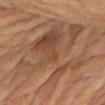<lesion>
<biopsy_status>not biopsied; imaged during a skin examination</biopsy_status>
<automated_metrics>
  <eccentricity>0.7</eccentricity>
  <cielab_L>46</cielab_L>
  <cielab_a>19</cielab_a>
  <cielab_b>30</cielab_b>
  <vs_skin_darker_L>7.0</vs_skin_darker_L>
  <vs_skin_contrast_norm>6.0</vs_skin_contrast_norm>
  <border_irregularity_0_10>9.0</border_irregularity_0_10>
  <color_variation_0_10>6.5</color_variation_0_10>
  <peripheral_color_asymmetry>2.0</peripheral_color_asymmetry>
  <nevus_likeness_0_100>25</nevus_likeness_0_100>
  <lesion_detection_confidence_0_100>95</lesion_detection_confidence_0_100>
</automated_metrics>
<image>
  <source>total-body photography crop</source>
  <field_of_view_mm>15</field_of_view_mm>
</image>
<lesion_size>
  <long_diameter_mm_approx>12.0</long_diameter_mm_approx>
</lesion_size>
<site>left upper arm</site>
<patient>
  <sex>female</sex>
  <age_approx>65</age_approx>
</patient>
</lesion>